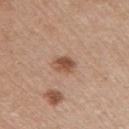Clinical impression:
No biopsy was performed on this lesion — it was imaged during a full skin examination and was not determined to be concerning.
Context:
On the right upper arm. The total-body-photography lesion software estimated a lesion area of about 4 mm², an outline eccentricity of about 0.65 (0 = round, 1 = elongated), and a shape-asymmetry score of about 0.25 (0 = symmetric). The analysis additionally found a lesion color around L≈51 a*≈21 b*≈31 in CIELAB, about 12 CIELAB-L* units darker than the surrounding skin, and a normalized lesion–skin contrast near 9. Longest diameter approximately 2.5 mm. A close-up tile cropped from a whole-body skin photograph, about 15 mm across. The patient is a female about 50 years old.